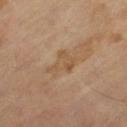Assessment: Recorded during total-body skin imaging; not selected for excision or biopsy. Context: From the left thigh. The lesion-visualizer software estimated an area of roughly 5.5 mm², an eccentricity of roughly 0.65, and a shape-asymmetry score of about 0.5 (0 = symmetric). It also reported a lesion color around L≈50 a*≈16 b*≈33 in CIELAB, about 6 CIELAB-L* units darker than the surrounding skin, and a lesion-to-skin contrast of about 5.5 (normalized; higher = more distinct). The analysis additionally found a border-irregularity index near 5.5/10, a color-variation rating of about 2.5/10, and peripheral color asymmetry of about 1. It also reported a detector confidence of about 100 out of 100 that the crop contains a lesion. Measured at roughly 3.5 mm in maximum diameter. A male patient, roughly 65 years of age. A 15 mm close-up tile from a total-body photography series done for melanoma screening.